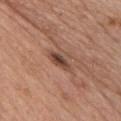Located on the chest. A male subject, in their mid-50s. Approximately 3.5 mm at its widest. Cropped from a total-body skin-imaging series; the visible field is about 15 mm. Imaged with white-light lighting.